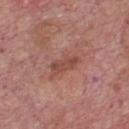Assessment: Imaged during a routine full-body skin examination; the lesion was not biopsied and no histopathology is available. Image and clinical context: Automated image analysis of the tile measured an area of roughly 5 mm², an eccentricity of roughly 0.9, and two-axis asymmetry of about 0.3. The software also gave a mean CIELAB color near L≈47 a*≈24 b*≈26 and about 9 CIELAB-L* units darker than the surrounding skin. The lesion's longest dimension is about 4 mm. The lesion is located on the chest. A male subject roughly 75 years of age. A lesion tile, about 15 mm wide, cut from a 3D total-body photograph. This is a white-light tile.